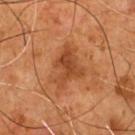Q: Was a biopsy performed?
A: imaged on a skin check; not biopsied
Q: Lesion size?
A: about 5.5 mm
Q: What is the anatomic site?
A: the chest
Q: What is the imaging modality?
A: total-body-photography crop, ~15 mm field of view
Q: Who is the patient?
A: male, aged 53–57
Q: Automated lesion metrics?
A: a footprint of about 12 mm² and a shape eccentricity near 0.75; peripheral color asymmetry of about 1.5; an automated nevus-likeness rating near 20 out of 100 and lesion-presence confidence of about 100/100